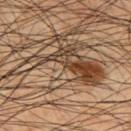Impression: This lesion was catalogued during total-body skin photography and was not selected for biopsy. Background: This image is a 15 mm lesion crop taken from a total-body photograph. A male patient in their 50s. On the upper back. This is a cross-polarized tile. About 11 mm across.